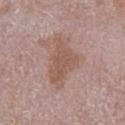Q: Was this lesion biopsied?
A: no biopsy performed (imaged during a skin exam)